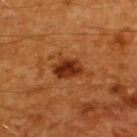No biopsy was performed on this lesion — it was imaged during a full skin examination and was not determined to be concerning. A male patient aged 58–62. A roughly 15 mm field-of-view crop from a total-body skin photograph. From the upper back.